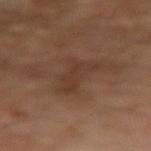Imaged during a routine full-body skin examination; the lesion was not biopsied and no histopathology is available.
Longest diameter approximately 4 mm.
A 15 mm close-up extracted from a 3D total-body photography capture.
From the right forearm.
This is a cross-polarized tile.
A male patient aged approximately 65.